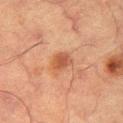Case summary:
* biopsy status — no biopsy performed (imaged during a skin exam)
* automated lesion analysis — an area of roughly 4 mm², an outline eccentricity of about 0.6 (0 = round, 1 = elongated), and two-axis asymmetry of about 0.25; internal color variation of about 2.5 on a 0–10 scale and peripheral color asymmetry of about 1
* tile lighting — cross-polarized illumination
* site — the left thigh
* imaging modality — 15 mm crop, total-body photography
* patient — male, in their mid-60s
* lesion size — about 2.5 mm